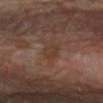biopsy status: imaged on a skin check; not biopsied | imaging modality: ~15 mm crop, total-body skin-cancer survey | image-analysis metrics: a footprint of about 4 mm², an outline eccentricity of about 0.8 (0 = round, 1 = elongated), and a shape-asymmetry score of about 0.3 (0 = symmetric); an average lesion color of about L≈37 a*≈18 b*≈26 (CIELAB) and a lesion–skin lightness drop of about 6; a border-irregularity index near 2.5/10, internal color variation of about 2 on a 0–10 scale, and peripheral color asymmetry of about 1 | site: the right forearm | size: ~3 mm (longest diameter) | subject: female, aged around 30 | illumination: cross-polarized.